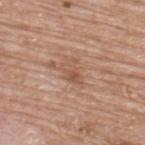Findings:
• notes — no biopsy performed (imaged during a skin exam)
• diameter — ~3 mm (longest diameter)
• subject — male, in their mid- to late 60s
• lighting — white-light illumination
• site — the back
• imaging modality — ~15 mm crop, total-body skin-cancer survey